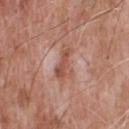notes = imaged on a skin check; not biopsied
patient = male, aged approximately 50
anatomic site = the back
image source = ~15 mm crop, total-body skin-cancer survey